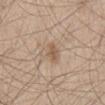{
  "biopsy_status": "not biopsied; imaged during a skin examination",
  "image": {
    "source": "total-body photography crop",
    "field_of_view_mm": 15
  },
  "lesion_size": {
    "long_diameter_mm_approx": 3.0
  },
  "site": "right thigh",
  "patient": {
    "sex": "male",
    "age_approx": 60
  }
}